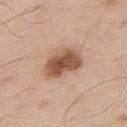Notes:
- workup — total-body-photography surveillance lesion; no biopsy
- lesion diameter — ~4.5 mm (longest diameter)
- acquisition — 15 mm crop, total-body photography
- patient — male, aged 53–57
- site — the back
- tile lighting — white-light illumination